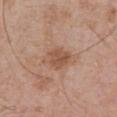* patient — male, aged 68 to 72
* lighting — white-light
* location — the abdomen
* image source — total-body-photography crop, ~15 mm field of view
* lesion diameter — ≈3.5 mm